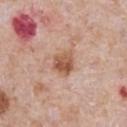* notes: no biopsy performed (imaged during a skin exam)
* acquisition: 15 mm crop, total-body photography
* patient: male, aged 58–62
* location: the front of the torso